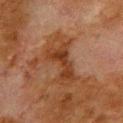Recorded during total-body skin imaging; not selected for excision or biopsy.
The patient is a male in their 80s.
Longest diameter approximately 7 mm.
On the back.
Imaged with cross-polarized lighting.
A lesion tile, about 15 mm wide, cut from a 3D total-body photograph.